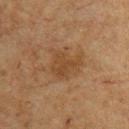Impression:
The lesion was tiled from a total-body skin photograph and was not biopsied.
Acquisition and patient details:
Captured under cross-polarized illumination. The lesion's longest dimension is about 4 mm. On the chest. This image is a 15 mm lesion crop taken from a total-body photograph. A male patient, in their mid- to late 60s.